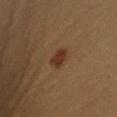| feature | finding |
|---|---|
| workup | catalogued during a skin exam; not biopsied |
| patient | female, aged 23–27 |
| diameter | ≈2.5 mm |
| TBP lesion metrics | a shape eccentricity near 0.75 and a symmetry-axis asymmetry near 0.2; a border-irregularity index near 2/10, a color-variation rating of about 2/10, and a peripheral color-asymmetry measure near 0.5 |
| acquisition | ~15 mm crop, total-body skin-cancer survey |
| anatomic site | the right upper arm |
| illumination | cross-polarized |